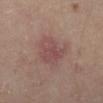Clinical impression: Imaged during a routine full-body skin examination; the lesion was not biopsied and no histopathology is available. Acquisition and patient details: A 15 mm crop from a total-body photograph taken for skin-cancer surveillance. The patient is a female about 35 years old. The lesion is on the left lower leg.